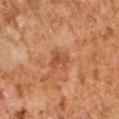Assessment: Part of a total-body skin-imaging series; this lesion was reviewed on a skin check and was not flagged for biopsy. Acquisition and patient details: A region of skin cropped from a whole-body photographic capture, roughly 15 mm wide. Captured under cross-polarized illumination. Automated tile analysis of the lesion measured a footprint of about 4 mm² and a shape-asymmetry score of about 0.3 (0 = symmetric). And it measured a lesion color around L≈47 a*≈26 b*≈33 in CIELAB, a lesion–skin lightness drop of about 8, and a normalized border contrast of about 6.5. It also reported a border-irregularity rating of about 3.5/10, a color-variation rating of about 2/10, and a peripheral color-asymmetry measure near 1. The lesion is on the right forearm. A male subject, roughly 60 years of age. The recorded lesion diameter is about 2.5 mm.